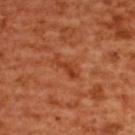notes=no biopsy performed (imaged during a skin exam); location=the upper back; subject=female, aged around 55; image=~15 mm crop, total-body skin-cancer survey.